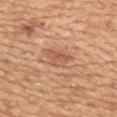Assessment: Captured during whole-body skin photography for melanoma surveillance; the lesion was not biopsied. Clinical summary: Captured under white-light illumination. A female patient, aged 53 to 57. From the upper back. A 15 mm close-up extracted from a 3D total-body photography capture.